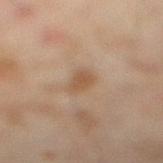  biopsy_status: not biopsied; imaged during a skin examination
  site: right lower leg
  image:
    source: total-body photography crop
    field_of_view_mm: 15
  lighting: cross-polarized
  automated_metrics:
    cielab_L: 44
    cielab_a: 15
    cielab_b: 28
    vs_skin_darker_L: 7.0
    vs_skin_contrast_norm: 6.5
    border_irregularity_0_10: 2.5
    color_variation_0_10: 1.5
    peripheral_color_asymmetry: 0.5
  patient:
    sex: male
    age_approx: 45